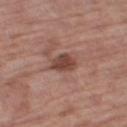Part of a total-body skin-imaging series; this lesion was reviewed on a skin check and was not flagged for biopsy. A roughly 15 mm field-of-view crop from a total-body skin photograph. A male subject, aged approximately 70. Located on the right thigh.